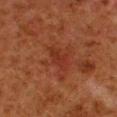workup: imaged on a skin check; not biopsied | illumination: cross-polarized illumination | patient: male, aged 78–82 | lesion diameter: ≈3.5 mm | TBP lesion metrics: a mean CIELAB color near L≈26 a*≈24 b*≈26; a border-irregularity rating of about 4.5/10, internal color variation of about 3 on a 0–10 scale, and a peripheral color-asymmetry measure near 1 | anatomic site: the left lower leg | image: ~15 mm crop, total-body skin-cancer survey.